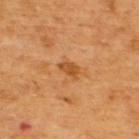Clinical impression: No biopsy was performed on this lesion — it was imaged during a full skin examination and was not determined to be concerning. Background: Imaged with cross-polarized lighting. A male subject, aged 63–67. Located on the upper back. About 2.5 mm across. A region of skin cropped from a whole-body photographic capture, roughly 15 mm wide.